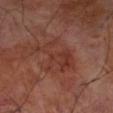Clinical impression: Part of a total-body skin-imaging series; this lesion was reviewed on a skin check and was not flagged for biopsy. Background: The patient is a male approximately 70 years of age. The lesion is on the left forearm. The tile uses cross-polarized illumination. A lesion tile, about 15 mm wide, cut from a 3D total-body photograph.